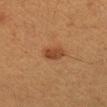* image-analysis metrics · about 9 CIELAB-L* units darker than the surrounding skin and a normalized lesion–skin contrast near 8; a border-irregularity rating of about 2/10, a color-variation rating of about 2.5/10, and a peripheral color-asymmetry measure near 1; an automated nevus-likeness rating near 90 out of 100 and a detector confidence of about 100 out of 100 that the crop contains a lesion
* lesion size · ~2.5 mm (longest diameter)
* anatomic site · the arm
* tile lighting · cross-polarized
* patient · female, aged approximately 40
* image source · ~15 mm crop, total-body skin-cancer survey